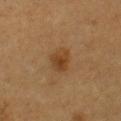Clinical impression:
The lesion was tiled from a total-body skin photograph and was not biopsied.
Context:
A close-up tile cropped from a whole-body skin photograph, about 15 mm across. The lesion is on the front of the torso. About 3 mm across. The patient is a male aged approximately 60. This is a cross-polarized tile.